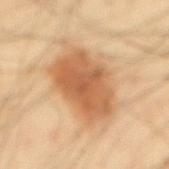Clinical summary:
The patient is a male aged approximately 40. A region of skin cropped from a whole-body photographic capture, roughly 15 mm wide. Imaged with cross-polarized lighting. Automated tile analysis of the lesion measured a lesion area of about 31 mm² and an outline eccentricity of about 0.7 (0 = round, 1 = elongated). The software also gave a border-irregularity rating of about 3/10, a color-variation rating of about 4.5/10, and a peripheral color-asymmetry measure near 1. Approximately 8.5 mm at its widest. On the mid back.